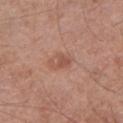Imaged during a routine full-body skin examination; the lesion was not biopsied and no histopathology is available. On the left lower leg. A male patient approximately 60 years of age. The lesion's longest dimension is about 3 mm. Automated image analysis of the tile measured an area of roughly 4 mm² and two-axis asymmetry of about 0.35. The tile uses white-light illumination. A 15 mm close-up extracted from a 3D total-body photography capture.